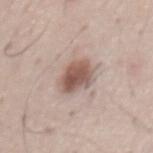<record>
<biopsy_status>not biopsied; imaged during a skin examination</biopsy_status>
<site>chest</site>
<patient>
  <sex>male</sex>
  <age_approx>60</age_approx>
</patient>
<image>
  <source>total-body photography crop</source>
  <field_of_view_mm>15</field_of_view_mm>
</image>
</record>